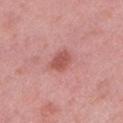Q: How was this image acquired?
A: ~15 mm crop, total-body skin-cancer survey
Q: Who is the patient?
A: female, approximately 40 years of age
Q: Automated lesion metrics?
A: a footprint of about 5 mm², an outline eccentricity of about 0.7 (0 = round, 1 = elongated), and a shape-asymmetry score of about 0.2 (0 = symmetric)
Q: Where on the body is the lesion?
A: the left lower leg
Q: What is the lesion's diameter?
A: ~3 mm (longest diameter)
Q: Illumination type?
A: white-light illumination